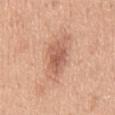  biopsy_status: not biopsied; imaged during a skin examination
  image:
    source: total-body photography crop
    field_of_view_mm: 15
  site: mid back
  patient:
    sex: male
    age_approx: 50
  lighting: white-light
  lesion_size:
    long_diameter_mm_approx: 7.0